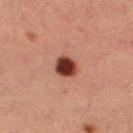Imaged during a routine full-body skin examination; the lesion was not biopsied and no histopathology is available. A female subject approximately 55 years of age. The lesion is located on the left thigh. Imaged with cross-polarized lighting. This image is a 15 mm lesion crop taken from a total-body photograph.